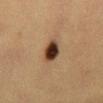The patient is a female in their 40s. The lesion-visualizer software estimated an area of roughly 6.5 mm², an outline eccentricity of about 0.7 (0 = round, 1 = elongated), and a shape-asymmetry score of about 0.15 (0 = symmetric). The analysis additionally found roughly 17 lightness units darker than nearby skin and a lesion-to-skin contrast of about 15 (normalized; higher = more distinct). And it measured a border-irregularity rating of about 1.5/10, a within-lesion color-variation index near 5/10, and radial color variation of about 1.5. On the abdomen. A close-up tile cropped from a whole-body skin photograph, about 15 mm across. The tile uses cross-polarized illumination.